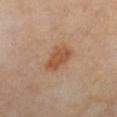Background:
Longest diameter approximately 3.5 mm. The lesion is located on the leg. A roughly 15 mm field-of-view crop from a total-body skin photograph. A male subject, about 55 years old.